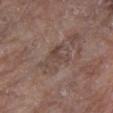notes: total-body-photography surveillance lesion; no biopsy
lesion diameter: ~4.5 mm (longest diameter)
lighting: white-light
location: the chest
TBP lesion metrics: a mean CIELAB color near L≈45 a*≈15 b*≈21 and a lesion-to-skin contrast of about 5 (normalized; higher = more distinct); an automated nevus-likeness rating near 0 out of 100
acquisition: ~15 mm crop, total-body skin-cancer survey
subject: female, about 75 years old